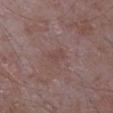Part of a total-body skin-imaging series; this lesion was reviewed on a skin check and was not flagged for biopsy. The lesion is located on the left upper arm. A 15 mm close-up tile from a total-body photography series done for melanoma screening. This is a white-light tile. The subject is a male aged 63 to 67. The lesion-visualizer software estimated an area of roughly 3 mm² and a symmetry-axis asymmetry near 0.25. It also reported an average lesion color of about L≈45 a*≈18 b*≈21 (CIELAB), a lesion–skin lightness drop of about 4, and a normalized border contrast of about 4.5. The analysis additionally found a classifier nevus-likeness of about 0/100 and a lesion-detection confidence of about 100/100.